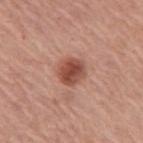Clinical impression:
Recorded during total-body skin imaging; not selected for excision or biopsy.
Background:
This image is a 15 mm lesion crop taken from a total-body photograph. The subject is a female in their mid- to late 60s. The lesion is located on the arm. The lesion's longest dimension is about 3 mm.